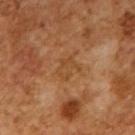The lesion was photographed on a routine skin check and not biopsied; there is no pathology result.
A male subject, about 65 years old.
A 15 mm close-up tile from a total-body photography series done for melanoma screening.
The total-body-photography lesion software estimated a footprint of about 5 mm². The analysis additionally found a normalized lesion–skin contrast near 5. The analysis additionally found a border-irregularity rating of about 5.5/10, internal color variation of about 2 on a 0–10 scale, and peripheral color asymmetry of about 0.5. The analysis additionally found a classifier nevus-likeness of about 0/100 and a detector confidence of about 100 out of 100 that the crop contains a lesion.